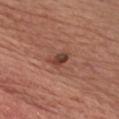{
  "biopsy_status": "not biopsied; imaged during a skin examination",
  "lighting": "white-light",
  "site": "front of the torso",
  "automated_metrics": {
    "area_mm2_approx": 3.5,
    "eccentricity": 0.8,
    "shape_asymmetry": 0.3,
    "cielab_L": 41,
    "cielab_a": 23,
    "cielab_b": 26,
    "vs_skin_darker_L": 11.0,
    "vs_skin_contrast_norm": 9.0,
    "border_irregularity_0_10": 3.0,
    "color_variation_0_10": 5.5,
    "peripheral_color_asymmetry": 1.5
  },
  "image": {
    "source": "total-body photography crop",
    "field_of_view_mm": 15
  },
  "patient": {
    "sex": "male",
    "age_approx": 60
  }
}